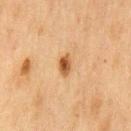Captured during whole-body skin photography for melanoma surveillance; the lesion was not biopsied. A 15 mm crop from a total-body photograph taken for skin-cancer surveillance. The total-body-photography lesion software estimated roughly 12 lightness units darker than nearby skin and a lesion-to-skin contrast of about 10 (normalized; higher = more distinct). On the chest. This is a cross-polarized tile. A male patient, in their mid- to late 70s.